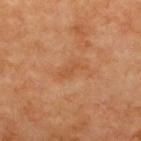workup: catalogued during a skin exam; not biopsied
illumination: cross-polarized
patient: in their mid-60s
location: the upper back
diameter: about 3 mm
automated metrics: a lesion–skin lightness drop of about 6 and a lesion-to-skin contrast of about 5 (normalized; higher = more distinct)
imaging modality: 15 mm crop, total-body photography